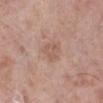Impression:
The lesion was tiled from a total-body skin photograph and was not biopsied.
Background:
The lesion's longest dimension is about 3 mm. An algorithmic analysis of the crop reported a nevus-likeness score of about 0/100 and a detector confidence of about 100 out of 100 that the crop contains a lesion. Cropped from a whole-body photographic skin survey; the tile spans about 15 mm. The lesion is located on the leg. The patient is a female aged approximately 70. The tile uses white-light illumination.